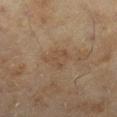- imaging modality — ~15 mm crop, total-body skin-cancer survey
- lighting — cross-polarized
- lesion diameter — ~3 mm (longest diameter)
- subject — female, aged around 60
- automated lesion analysis — a lesion area of about 5 mm²; a mean CIELAB color near L≈43 a*≈14 b*≈28, a lesion–skin lightness drop of about 5, and a normalized lesion–skin contrast near 4.5; border irregularity of about 3.5 on a 0–10 scale, a color-variation rating of about 1.5/10, and radial color variation of about 0.5
- body site — the leg